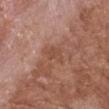Assessment:
Part of a total-body skin-imaging series; this lesion was reviewed on a skin check and was not flagged for biopsy.
Image and clinical context:
Longest diameter approximately 3 mm. A male subject, in their mid- to late 60s. The lesion-visualizer software estimated a classifier nevus-likeness of about 0/100 and a detector confidence of about 100 out of 100 that the crop contains a lesion. From the left forearm. A region of skin cropped from a whole-body photographic capture, roughly 15 mm wide. The tile uses white-light illumination.